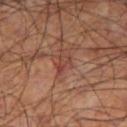Notes:
– follow-up · catalogued during a skin exam; not biopsied
– imaging modality · ~15 mm tile from a whole-body skin photo
– image-analysis metrics · an outline eccentricity of about 0.85 (0 = round, 1 = elongated); border irregularity of about 4 on a 0–10 scale and a within-lesion color-variation index near 0/10
– subject · male, aged 58 to 62
– location · the right thigh
– lesion size · about 2.5 mm